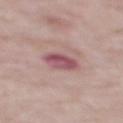workup = catalogued during a skin exam; not biopsied | subject = male, approximately 65 years of age | size = about 3.5 mm | TBP lesion metrics = a shape eccentricity near 0.65 and a symmetry-axis asymmetry near 0.15; a lesion color around L≈52 a*≈26 b*≈16 in CIELAB, a lesion–skin lightness drop of about 12, and a normalized border contrast of about 9; border irregularity of about 1.5 on a 0–10 scale and internal color variation of about 5.5 on a 0–10 scale; an automated nevus-likeness rating near 0 out of 100 and a lesion-detection confidence of about 100/100 | body site = the mid back | tile lighting = white-light illumination | image = ~15 mm tile from a whole-body skin photo.